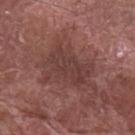Q: Is there a histopathology result?
A: catalogued during a skin exam; not biopsied
Q: What are the patient's age and sex?
A: male, in their mid-70s
Q: What is the imaging modality?
A: 15 mm crop, total-body photography
Q: Where on the body is the lesion?
A: the left forearm